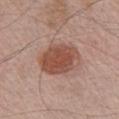<lesion>
  <biopsy_status>not biopsied; imaged during a skin examination</biopsy_status>
  <image>
    <source>total-body photography crop</source>
    <field_of_view_mm>15</field_of_view_mm>
  </image>
  <site>chest</site>
  <lighting>white-light</lighting>
  <lesion_size>
    <long_diameter_mm_approx>5.5</long_diameter_mm_approx>
  </lesion_size>
  <patient>
    <sex>male</sex>
    <age_approx>65</age_approx>
  </patient>
</lesion>